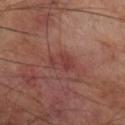image source: total-body-photography crop, ~15 mm field of view; site: the right lower leg; lesion diameter: about 3 mm; illumination: cross-polarized; patient: male, aged around 70.